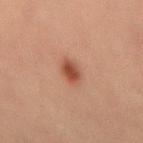Part of a total-body skin-imaging series; this lesion was reviewed on a skin check and was not flagged for biopsy. This is a cross-polarized tile. The lesion is located on the lower back. A male subject aged approximately 30. A close-up tile cropped from a whole-body skin photograph, about 15 mm across.